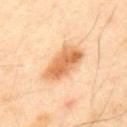- notes: imaged on a skin check; not biopsied
- anatomic site: the mid back
- image-analysis metrics: an eccentricity of roughly 0.9 and two-axis asymmetry of about 0.2; a lesion color around L≈68 a*≈25 b*≈42 in CIELAB and a normalized lesion–skin contrast near 9; a within-lesion color-variation index near 5.5/10
- lesion diameter: ~5.5 mm (longest diameter)
- patient: male, about 50 years old
- imaging modality: ~15 mm crop, total-body skin-cancer survey